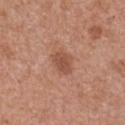The lesion was photographed on a routine skin check and not biopsied; there is no pathology result. The lesion's longest dimension is about 3 mm. From the front of the torso. Cropped from a total-body skin-imaging series; the visible field is about 15 mm. Captured under white-light illumination. A female patient aged 48–52. Automated image analysis of the tile measured about 9 CIELAB-L* units darker than the surrounding skin. And it measured a color-variation rating of about 1.5/10 and radial color variation of about 0.5. The analysis additionally found an automated nevus-likeness rating near 50 out of 100 and lesion-presence confidence of about 100/100.